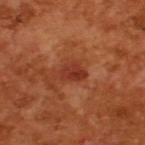| field | value |
|---|---|
| follow-up | total-body-photography surveillance lesion; no biopsy |
| subject | male, in their mid- to late 60s |
| image source | ~15 mm crop, total-body skin-cancer survey |